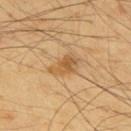The lesion was photographed on a routine skin check and not biopsied; there is no pathology result.
The subject is a male about 65 years old.
The lesion is located on the upper back.
A 15 mm close-up extracted from a 3D total-body photography capture.
Imaged with cross-polarized lighting.